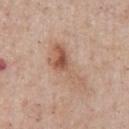Q: Is there a histopathology result?
A: catalogued during a skin exam; not biopsied
Q: What kind of image is this?
A: ~15 mm crop, total-body skin-cancer survey
Q: How was the tile lit?
A: white-light
Q: What did automated image analysis measure?
A: a normalized border contrast of about 7; internal color variation of about 7 on a 0–10 scale and a peripheral color-asymmetry measure near 2; a nevus-likeness score of about 50/100 and a lesion-detection confidence of about 100/100
Q: What are the patient's age and sex?
A: male, in their mid- to late 50s
Q: What is the anatomic site?
A: the chest
Q: How large is the lesion?
A: ≈6.5 mm